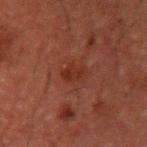biopsy_status: not biopsied; imaged during a skin examination
site: arm
image:
  source: total-body photography crop
  field_of_view_mm: 15
patient:
  sex: male
  age_approx: 50